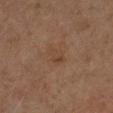Assessment:
Recorded during total-body skin imaging; not selected for excision or biopsy.
Image and clinical context:
Cropped from a total-body skin-imaging series; the visible field is about 15 mm. About 3 mm across. The tile uses cross-polarized illumination. The patient is a male aged around 85 or older. On the right lower leg.